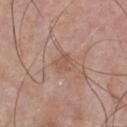{"automated_metrics": {"border_irregularity_0_10": 2.5, "color_variation_0_10": 1.5, "peripheral_color_asymmetry": 0.5}, "lesion_size": {"long_diameter_mm_approx": 2.5}, "lighting": "white-light", "patient": {"sex": "male", "age_approx": 55}, "site": "chest", "image": {"source": "total-body photography crop", "field_of_view_mm": 15}}